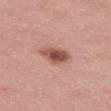Recorded during total-body skin imaging; not selected for excision or biopsy.
A lesion tile, about 15 mm wide, cut from a 3D total-body photograph.
A female subject, roughly 45 years of age.
The lesion-visualizer software estimated a mean CIELAB color near L≈53 a*≈24 b*≈26, roughly 14 lightness units darker than nearby skin, and a normalized lesion–skin contrast near 9. The analysis additionally found a border-irregularity index near 2/10, internal color variation of about 5.5 on a 0–10 scale, and peripheral color asymmetry of about 1.5.
The lesion is on the left thigh.
Approximately 4 mm at its widest.
Captured under white-light illumination.